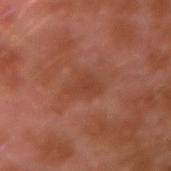{
  "site": "left arm",
  "image": {
    "source": "total-body photography crop",
    "field_of_view_mm": 15
  },
  "patient": {
    "sex": "male",
    "age_approx": 30
  }
}